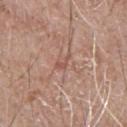Q: Is there a histopathology result?
A: catalogued during a skin exam; not biopsied
Q: What kind of image is this?
A: ~15 mm crop, total-body skin-cancer survey
Q: What is the anatomic site?
A: the chest
Q: Who is the patient?
A: male, in their mid-60s